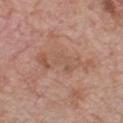Assessment:
The lesion was tiled from a total-body skin photograph and was not biopsied.
Context:
A roughly 15 mm field-of-view crop from a total-body skin photograph. Measured at roughly 7 mm in maximum diameter. Imaged with white-light lighting. The lesion is on the front of the torso. Automated image analysis of the tile measured a lesion area of about 12 mm², an eccentricity of roughly 0.95, and a shape-asymmetry score of about 0.45 (0 = symmetric). The analysis additionally found a lesion–skin lightness drop of about 7 and a lesion-to-skin contrast of about 5 (normalized; higher = more distinct). A male patient, approximately 60 years of age.